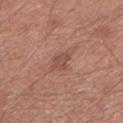biopsy status: no biopsy performed (imaged during a skin exam)
subject: male, aged approximately 65
site: the left lower leg
image: total-body-photography crop, ~15 mm field of view